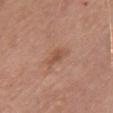notes — catalogued during a skin exam; not biopsied | body site — the chest | TBP lesion metrics — an average lesion color of about L≈52 a*≈22 b*≈31 (CIELAB) and about 8 CIELAB-L* units darker than the surrounding skin; a lesion-detection confidence of about 100/100 | subject — female, aged approximately 50 | image source — ~15 mm tile from a whole-body skin photo.